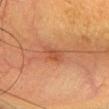No biopsy was performed on this lesion — it was imaged during a full skin examination and was not determined to be concerning. A female subject in their mid-50s. Located on the head or neck. This is a cross-polarized tile. A 15 mm close-up extracted from a 3D total-body photography capture. The lesion's longest dimension is about 2.5 mm. Automated image analysis of the tile measured a lesion area of about 3.5 mm², an eccentricity of roughly 0.75, and two-axis asymmetry of about 0.35. And it measured a lesion color around L≈43 a*≈24 b*≈32 in CIELAB, a lesion–skin lightness drop of about 7, and a normalized lesion–skin contrast near 6. The software also gave an automated nevus-likeness rating near 20 out of 100 and lesion-presence confidence of about 100/100.